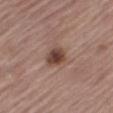workup: total-body-photography surveillance lesion; no biopsy | image source: total-body-photography crop, ~15 mm field of view | patient: male, about 65 years old | size: ≈3 mm | location: the right thigh | automated lesion analysis: a footprint of about 5.5 mm², an eccentricity of roughly 0.65, and a symmetry-axis asymmetry near 0.2; an average lesion color of about L≈42 a*≈19 b*≈25 (CIELAB), roughly 13 lightness units darker than nearby skin, and a lesion-to-skin contrast of about 10.5 (normalized; higher = more distinct); a border-irregularity rating of about 1.5/10 and a within-lesion color-variation index near 4.5/10; a classifier nevus-likeness of about 90/100 and a lesion-detection confidence of about 100/100 | lighting: white-light illumination.